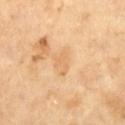Assessment: Imaged during a routine full-body skin examination; the lesion was not biopsied and no histopathology is available. Acquisition and patient details: Imaged with cross-polarized lighting. Cropped from a whole-body photographic skin survey; the tile spans about 15 mm. A male subject, roughly 65 years of age.